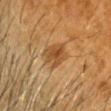notes: catalogued during a skin exam; not biopsied | lesion size: ≈3.5 mm | body site: the head or neck | subject: male, in their 60s | illumination: cross-polarized illumination | automated metrics: a lesion area of about 8 mm², an outline eccentricity of about 0.5 (0 = round, 1 = elongated), and a shape-asymmetry score of about 0.2 (0 = symmetric); a nevus-likeness score of about 65/100 and a detector confidence of about 100 out of 100 that the crop contains a lesion | imaging modality: ~15 mm tile from a whole-body skin photo.